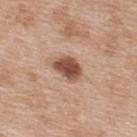lighting: white-light illumination
subject: female, approximately 40 years of age
acquisition: ~15 mm tile from a whole-body skin photo
body site: the upper back
diameter: about 3.5 mm
TBP lesion metrics: a lesion area of about 7 mm², an eccentricity of roughly 0.7, and two-axis asymmetry of about 0.25; a mean CIELAB color near L≈50 a*≈21 b*≈29, a lesion–skin lightness drop of about 17, and a normalized lesion–skin contrast near 11; an automated nevus-likeness rating near 90 out of 100 and lesion-presence confidence of about 100/100